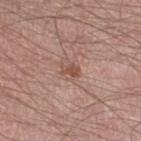| key | value |
|---|---|
| biopsy status | imaged on a skin check; not biopsied |
| image-analysis metrics | a lesion area of about 3.5 mm² and a shape-asymmetry score of about 0.25 (0 = symmetric); an average lesion color of about L≈52 a*≈21 b*≈26 (CIELAB), roughly 8 lightness units darker than nearby skin, and a normalized lesion–skin contrast near 6.5; a border-irregularity rating of about 3/10 and a peripheral color-asymmetry measure near 0.5 |
| body site | the leg |
| image | 15 mm crop, total-body photography |
| subject | male, aged 33–37 |